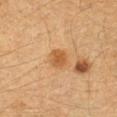Imaged during a routine full-body skin examination; the lesion was not biopsied and no histopathology is available.
Automated tile analysis of the lesion measured a lesion area of about 5 mm², an eccentricity of roughly 0.5, and a shape-asymmetry score of about 0.15 (0 = symmetric).
The patient is a female aged around 40.
The lesion is located on the left forearm.
A 15 mm crop from a total-body photograph taken for skin-cancer surveillance.
The recorded lesion diameter is about 2.5 mm.
Captured under cross-polarized illumination.